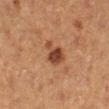No biopsy was performed on this lesion — it was imaged during a full skin examination and was not determined to be concerning. This is a cross-polarized tile. A female subject in their 40s. This image is a 15 mm lesion crop taken from a total-body photograph. From the right lower leg. Approximately 3.5 mm at its widest. An algorithmic analysis of the crop reported a lesion color around L≈36 a*≈21 b*≈28 in CIELAB and a normalized lesion–skin contrast near 9.5.